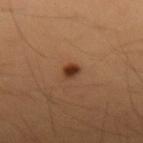Findings:
* biopsy status: no biopsy performed (imaged during a skin exam)
* acquisition: ~15 mm crop, total-body skin-cancer survey
* image-analysis metrics: a footprint of about 3 mm², a shape eccentricity near 0.35, and two-axis asymmetry of about 0.2; an average lesion color of about L≈36 a*≈22 b*≈32 (CIELAB) and a lesion–skin lightness drop of about 13
* body site: the left forearm
* patient: female, about 40 years old
* lighting: cross-polarized